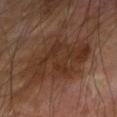{
  "biopsy_status": "not biopsied; imaged during a skin examination",
  "image": {
    "source": "total-body photography crop",
    "field_of_view_mm": 15
  },
  "lesion_size": {
    "long_diameter_mm_approx": 7.0
  },
  "automated_metrics": {
    "cielab_L": 32,
    "cielab_a": 18,
    "cielab_b": 27,
    "vs_skin_darker_L": 7.0,
    "vs_skin_contrast_norm": 7.0,
    "border_irregularity_0_10": 6.0,
    "peripheral_color_asymmetry": 1.5,
    "nevus_likeness_0_100": 5
  },
  "lighting": "cross-polarized",
  "site": "right forearm",
  "patient": {
    "sex": "male",
    "age_approx": 70
  }
}